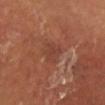Q: Is there a histopathology result?
A: imaged on a skin check; not biopsied
Q: How was this image acquired?
A: ~15 mm crop, total-body skin-cancer survey
Q: What is the anatomic site?
A: the leg
Q: How was the tile lit?
A: cross-polarized illumination
Q: What are the patient's age and sex?
A: male, in their mid-50s
Q: Lesion size?
A: ≈3 mm